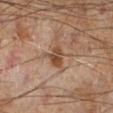Q: What lighting was used for the tile?
A: cross-polarized illumination
Q: What is the imaging modality?
A: 15 mm crop, total-body photography
Q: Lesion location?
A: the left leg
Q: What are the patient's age and sex?
A: male, in their 60s
Q: Automated lesion metrics?
A: an area of roughly 4.5 mm², a shape eccentricity near 0.65, and two-axis asymmetry of about 0.3; an average lesion color of about L≈34 a*≈15 b*≈24 (CIELAB), about 9 CIELAB-L* units darker than the surrounding skin, and a lesion-to-skin contrast of about 8.5 (normalized; higher = more distinct); a border-irregularity index near 3/10, a within-lesion color-variation index near 5/10, and radial color variation of about 2